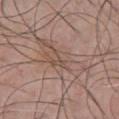Q: Was a biopsy performed?
A: catalogued during a skin exam; not biopsied
Q: What did automated image analysis measure?
A: an area of roughly 16 mm², a shape eccentricity near 0.9, and a shape-asymmetry score of about 0.45 (0 = symmetric); border irregularity of about 7 on a 0–10 scale, internal color variation of about 5.5 on a 0–10 scale, and radial color variation of about 2
Q: Lesion size?
A: ~7.5 mm (longest diameter)
Q: What lighting was used for the tile?
A: white-light
Q: What is the imaging modality?
A: 15 mm crop, total-body photography
Q: Who is the patient?
A: male, aged around 45
Q: What is the anatomic site?
A: the chest